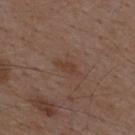Q: Was a biopsy performed?
A: catalogued during a skin exam; not biopsied
Q: What kind of image is this?
A: 15 mm crop, total-body photography
Q: What is the anatomic site?
A: the mid back
Q: Patient demographics?
A: male, about 50 years old